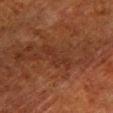workup=catalogued during a skin exam; not biopsied | image-analysis metrics=a lesion area of about 5 mm², a shape eccentricity near 0.95, and a symmetry-axis asymmetry near 0.7; a classifier nevus-likeness of about 0/100 and a detector confidence of about 70 out of 100 that the crop contains a lesion | acquisition=total-body-photography crop, ~15 mm field of view | site=the left lower leg | subject=male, approximately 80 years of age | lesion size=~4.5 mm (longest diameter) | tile lighting=cross-polarized.